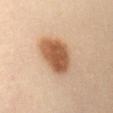<tbp_lesion>
  <automated_metrics>
    <area_mm2_approx>15.0</area_mm2_approx>
    <eccentricity>0.7</eccentricity>
    <shape_asymmetry>0.2</shape_asymmetry>
    <color_variation_0_10>4.5</color_variation_0_10>
    <peripheral_color_asymmetry>1.5</peripheral_color_asymmetry>
    <nevus_likeness_0_100>100</nevus_likeness_0_100>
  </automated_metrics>
  <image>
    <source>total-body photography crop</source>
    <field_of_view_mm>15</field_of_view_mm>
  </image>
  <lesion_size>
    <long_diameter_mm_approx>5.5</long_diameter_mm_approx>
  </lesion_size>
  <site>abdomen</site>
  <lighting>cross-polarized</lighting>
  <patient>
    <sex>female</sex>
    <age_approx>40</age_approx>
  </patient>
</tbp_lesion>